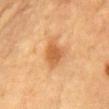Context: The lesion is located on the front of the torso. A male patient in their mid- to late 80s or older. A close-up tile cropped from a whole-body skin photograph, about 15 mm across.